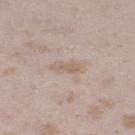Recorded during total-body skin imaging; not selected for excision or biopsy.
Located on the right thigh.
A female subject, about 25 years old.
This image is a 15 mm lesion crop taken from a total-body photograph.